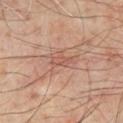Part of a total-body skin-imaging series; this lesion was reviewed on a skin check and was not flagged for biopsy.
Approximately 2.5 mm at its widest.
Cropped from a whole-body photographic skin survey; the tile spans about 15 mm.
A patient approximately 65 years of age.
On the front of the torso.
Captured under cross-polarized illumination.